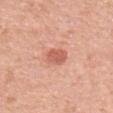Captured during whole-body skin photography for melanoma surveillance; the lesion was not biopsied.
Cropped from a total-body skin-imaging series; the visible field is about 15 mm.
A female subject, in their mid-50s.
Imaged with white-light lighting.
Longest diameter approximately 3 mm.
The lesion is located on the upper back.
The lesion-visualizer software estimated a border-irregularity rating of about 2/10 and radial color variation of about 0.5.